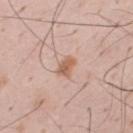workup=imaged on a skin check; not biopsied | subject=male, roughly 35 years of age | location=the mid back | acquisition=total-body-photography crop, ~15 mm field of view | tile lighting=white-light illumination.